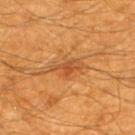{
  "biopsy_status": "not biopsied; imaged during a skin examination",
  "automated_metrics": {
    "area_mm2_approx": 3.5,
    "eccentricity": 0.85,
    "shape_asymmetry": 0.5
  },
  "lesion_size": {
    "long_diameter_mm_approx": 3.0
  },
  "patient": {
    "sex": "male",
    "age_approx": 60
  },
  "lighting": "cross-polarized",
  "site": "upper back",
  "image": {
    "source": "total-body photography crop",
    "field_of_view_mm": 15
  }
}